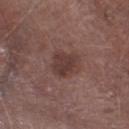Captured under white-light illumination. The lesion is on the right lower leg. A close-up tile cropped from a whole-body skin photograph, about 15 mm across. The recorded lesion diameter is about 3 mm. A male patient aged 73–77. The total-body-photography lesion software estimated a lesion area of about 7.5 mm², an eccentricity of roughly 0.4, and a symmetry-axis asymmetry near 0.25. The software also gave internal color variation of about 2.5 on a 0–10 scale and a peripheral color-asymmetry measure near 1.